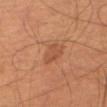The patient is a male approximately 60 years of age.
About 3 mm across.
From the right thigh.
Cropped from a whole-body photographic skin survey; the tile spans about 15 mm.